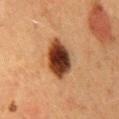Notes:
– biopsy status: no biopsy performed (imaged during a skin exam)
– location: the mid back
– subject: female, aged around 50
– acquisition: ~15 mm tile from a whole-body skin photo
– tile lighting: cross-polarized